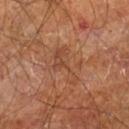Impression:
No biopsy was performed on this lesion — it was imaged during a full skin examination and was not determined to be concerning.
Acquisition and patient details:
From the right leg. A lesion tile, about 15 mm wide, cut from a 3D total-body photograph. This is a cross-polarized tile. A male patient aged approximately 60.